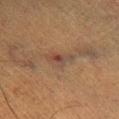Q: Was a biopsy performed?
A: catalogued during a skin exam; not biopsied
Q: How large is the lesion?
A: ~4 mm (longest diameter)
Q: What is the imaging modality?
A: ~15 mm tile from a whole-body skin photo
Q: What is the anatomic site?
A: the right lower leg
Q: Illumination type?
A: cross-polarized
Q: Patient demographics?
A: male, approximately 50 years of age